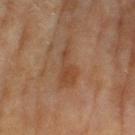notes = catalogued during a skin exam; not biopsied
patient = female, roughly 60 years of age
image source = ~15 mm tile from a whole-body skin photo
body site = the leg
lesion diameter = ~6 mm (longest diameter)